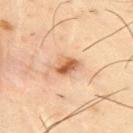notes = total-body-photography surveillance lesion; no biopsy
size = about 3 mm
tile lighting = cross-polarized
TBP lesion metrics = a nevus-likeness score of about 80/100 and a lesion-detection confidence of about 100/100
image source = ~15 mm tile from a whole-body skin photo
location = the right upper arm
subject = male, aged approximately 40